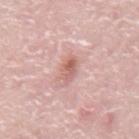A lesion tile, about 15 mm wide, cut from a 3D total-body photograph. On the back. The subject is a male roughly 75 years of age. Approximately 3 mm at its widest.